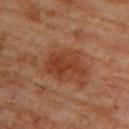The lesion was photographed on a routine skin check and not biopsied; there is no pathology result. Longest diameter approximately 5 mm. Imaged with cross-polarized lighting. Automated image analysis of the tile measured a mean CIELAB color near L≈39 a*≈26 b*≈33, a lesion–skin lightness drop of about 9, and a normalized lesion–skin contrast near 7.5. The software also gave border irregularity of about 4 on a 0–10 scale, internal color variation of about 3.5 on a 0–10 scale, and a peripheral color-asymmetry measure near 1. The software also gave an automated nevus-likeness rating near 95 out of 100 and a lesion-detection confidence of about 100/100. A female subject, aged 63–67. Located on the upper back. A region of skin cropped from a whole-body photographic capture, roughly 15 mm wide.